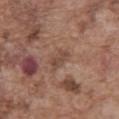Findings:
– image · ~15 mm crop, total-body skin-cancer survey
– illumination · white-light
– automated lesion analysis · a footprint of about 4 mm², a shape eccentricity near 0.85, and a symmetry-axis asymmetry near 0.2; a mean CIELAB color near L≈46 a*≈18 b*≈27 and a normalized lesion–skin contrast near 6; a color-variation rating of about 2/10
– patient · male, approximately 75 years of age
– location · the front of the torso